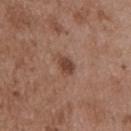Impression: Recorded during total-body skin imaging; not selected for excision or biopsy. Image and clinical context: The recorded lesion diameter is about 3 mm. The patient is a male approximately 50 years of age. From the back. Imaged with white-light lighting. Automated tile analysis of the lesion measured a footprint of about 4.5 mm², an eccentricity of roughly 0.8, and two-axis asymmetry of about 0.3. The analysis additionally found a lesion color around L≈44 a*≈20 b*≈27 in CIELAB, about 10 CIELAB-L* units darker than the surrounding skin, and a normalized lesion–skin contrast near 7.5. And it measured a border-irregularity index near 3.5/10, a color-variation rating of about 2/10, and radial color variation of about 0.5. It also reported an automated nevus-likeness rating near 90 out of 100 and a lesion-detection confidence of about 100/100. This image is a 15 mm lesion crop taken from a total-body photograph.